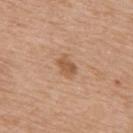Recorded during total-body skin imaging; not selected for excision or biopsy. Located on the upper back. The patient is a female aged around 65. A 15 mm crop from a total-body photograph taken for skin-cancer surveillance. An algorithmic analysis of the crop reported an area of roughly 4.5 mm², an outline eccentricity of about 0.75 (0 = round, 1 = elongated), and a shape-asymmetry score of about 0.2 (0 = symmetric). This is a white-light tile. The recorded lesion diameter is about 3 mm.